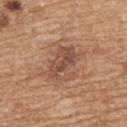biopsy status: catalogued during a skin exam; not biopsied
subject: female, approximately 70 years of age
location: the upper back
imaging modality: ~15 mm crop, total-body skin-cancer survey
lighting: white-light